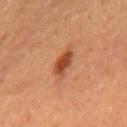{"biopsy_status": "not biopsied; imaged during a skin examination", "site": "back", "image": {"source": "total-body photography crop", "field_of_view_mm": 15}, "patient": {"sex": "male", "age_approx": 65}}